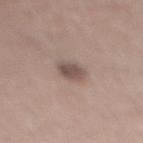| key | value |
|---|---|
| patient | female, aged around 70 |
| acquisition | ~15 mm tile from a whole-body skin photo |
| location | the right lower leg |
| lesion diameter | ~2.5 mm (longest diameter) |
| tile lighting | white-light |